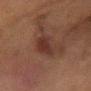biopsy status: no biopsy performed (imaged during a skin exam)
body site: the right forearm
lesion diameter: ~3.5 mm (longest diameter)
subject: female, in their 50s
lighting: cross-polarized
image: 15 mm crop, total-body photography
automated lesion analysis: a lesion area of about 8.5 mm² and two-axis asymmetry of about 0.25; a mean CIELAB color near L≈27 a*≈17 b*≈20 and roughly 6 lightness units darker than nearby skin; a classifier nevus-likeness of about 25/100